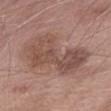{
  "biopsy_status": "not biopsied; imaged during a skin examination",
  "patient": {
    "sex": "female",
    "age_approx": 75
  },
  "automated_metrics": {
    "area_mm2_approx": 25.0,
    "eccentricity": 0.85,
    "shape_asymmetry": 0.3
  },
  "image": {
    "source": "total-body photography crop",
    "field_of_view_mm": 15
  },
  "lesion_size": {
    "long_diameter_mm_approx": 8.0
  },
  "site": "right thigh"
}